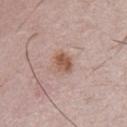Assessment:
Recorded during total-body skin imaging; not selected for excision or biopsy.
Image and clinical context:
The subject is a male aged 63 to 67. The lesion's longest dimension is about 3 mm. This is a white-light tile. The lesion is on the abdomen. Automated image analysis of the tile measured a lesion color around L≈55 a*≈20 b*≈27 in CIELAB and a normalized border contrast of about 8.5. The analysis additionally found a border-irregularity index near 2/10, a color-variation rating of about 3.5/10, and peripheral color asymmetry of about 1. A 15 mm crop from a total-body photograph taken for skin-cancer surveillance.